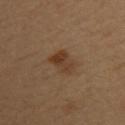Q: Was this lesion biopsied?
A: catalogued during a skin exam; not biopsied
Q: Who is the patient?
A: female, about 35 years old
Q: How was the tile lit?
A: cross-polarized illumination
Q: What is the anatomic site?
A: the upper back
Q: How was this image acquired?
A: 15 mm crop, total-body photography
Q: What is the lesion's diameter?
A: ~3 mm (longest diameter)